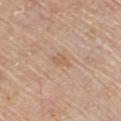- biopsy status · imaged on a skin check; not biopsied
- illumination · white-light
- lesion diameter · about 2.5 mm
- TBP lesion metrics · an average lesion color of about L≈60 a*≈18 b*≈32 (CIELAB), a lesion–skin lightness drop of about 7, and a normalized lesion–skin contrast near 5.5; internal color variation of about 1 on a 0–10 scale and peripheral color asymmetry of about 0.5
- site · the front of the torso
- subject · male, aged 78–82
- imaging modality · total-body-photography crop, ~15 mm field of view